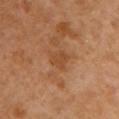Impression:
This lesion was catalogued during total-body skin photography and was not selected for biopsy.
Clinical summary:
From the chest. The subject is a female about 55 years old. A 15 mm crop from a total-body photograph taken for skin-cancer surveillance.